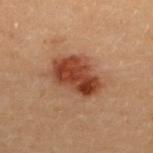follow-up = imaged on a skin check; not biopsied
location = the back
subject = female, aged 28 to 32
image = ~15 mm tile from a whole-body skin photo